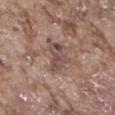biopsy_status: not biopsied; imaged during a skin examination
patient:
  sex: male
  age_approx: 75
site: leg
automated_metrics:
  cielab_L: 48
  cielab_a: 16
  cielab_b: 21
  vs_skin_darker_L: 9.0
  vs_skin_contrast_norm: 7.0
  border_irregularity_0_10: 4.5
  color_variation_0_10: 4.5
  peripheral_color_asymmetry: 1.5
  nevus_likeness_0_100: 0
  lesion_detection_confidence_0_100: 80
lighting: white-light
lesion_size:
  long_diameter_mm_approx: 4.0
image:
  source: total-body photography crop
  field_of_view_mm: 15